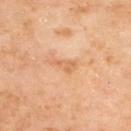Captured during whole-body skin photography for melanoma surveillance; the lesion was not biopsied. The lesion's longest dimension is about 3 mm. Located on the upper back. An algorithmic analysis of the crop reported an average lesion color of about L≈65 a*≈23 b*≈40 (CIELAB), roughly 8 lightness units darker than nearby skin, and a normalized border contrast of about 5.5. A female subject, roughly 60 years of age. A lesion tile, about 15 mm wide, cut from a 3D total-body photograph. The tile uses cross-polarized illumination.